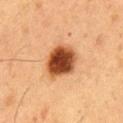follow-up: no biopsy performed (imaged during a skin exam)
subject: male, in their mid- to late 50s
diameter: about 4.5 mm
lighting: cross-polarized
image: total-body-photography crop, ~15 mm field of view
TBP lesion metrics: an area of roughly 14 mm² and an eccentricity of roughly 0.55; an average lesion color of about L≈44 a*≈25 b*≈36 (CIELAB), roughly 20 lightness units darker than nearby skin, and a lesion-to-skin contrast of about 13.5 (normalized; higher = more distinct); lesion-presence confidence of about 100/100
body site: the abdomen